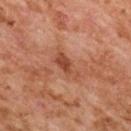follow-up=no biopsy performed (imaged during a skin exam) | anatomic site=the upper back | image source=total-body-photography crop, ~15 mm field of view | subject=female, in their 60s | illumination=cross-polarized illumination.